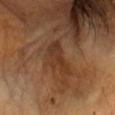The lesion was photographed on a routine skin check and not biopsied; there is no pathology result.
Cropped from a whole-body photographic skin survey; the tile spans about 15 mm.
The lesion is located on the head or neck.
The total-body-photography lesion software estimated radial color variation of about 1.5. The software also gave an automated nevus-likeness rating near 0 out of 100 and a lesion-detection confidence of about 80/100.
A male patient, in their mid-60s.
Longest diameter approximately 4.5 mm.
Captured under cross-polarized illumination.